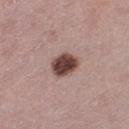workup: imaged on a skin check; not biopsied
image source: 15 mm crop, total-body photography
patient: female, aged 43 to 47
body site: the left thigh